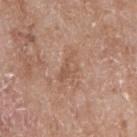Q: Was a biopsy performed?
A: no biopsy performed (imaged during a skin exam)
Q: What are the patient's age and sex?
A: female, in their mid- to late 70s
Q: What is the anatomic site?
A: the right upper arm
Q: How large is the lesion?
A: ≈4 mm
Q: How was this image acquired?
A: 15 mm crop, total-body photography
Q: What did automated image analysis measure?
A: a lesion color around L≈55 a*≈20 b*≈30 in CIELAB, about 7 CIELAB-L* units darker than the surrounding skin, and a normalized border contrast of about 5; a border-irregularity index near 5/10 and a within-lesion color-variation index near 3.5/10